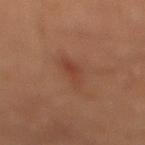Notes:
- biopsy status · imaged on a skin check; not biopsied
- size · ~4.5 mm (longest diameter)
- anatomic site · the mid back
- subject · male, aged approximately 60
- image · ~15 mm tile from a whole-body skin photo
- illumination · cross-polarized